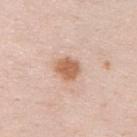Findings:
• biopsy status: no biopsy performed (imaged during a skin exam)
• imaging modality: total-body-photography crop, ~15 mm field of view
• lesion size: ~3 mm (longest diameter)
• subject: male, aged 33–37
• site: the back